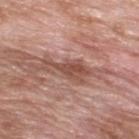Q: What is the imaging modality?
A: ~15 mm crop, total-body skin-cancer survey
Q: What is the anatomic site?
A: the upper back
Q: How large is the lesion?
A: ≈5.5 mm
Q: What are the patient's age and sex?
A: male, about 60 years old